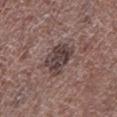notes = imaged on a skin check; not biopsied | anatomic site = the left lower leg | acquisition = total-body-photography crop, ~15 mm field of view | patient = male, in their 70s.